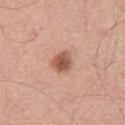follow-up: catalogued during a skin exam; not biopsied
image-analysis metrics: an area of roughly 5 mm², a shape eccentricity near 0.35, and a shape-asymmetry score of about 0.25 (0 = symmetric); a border-irregularity rating of about 2/10 and a within-lesion color-variation index near 5/10
lesion diameter: ≈2.5 mm
acquisition: ~15 mm crop, total-body skin-cancer survey
site: the right thigh
subject: male, aged 68–72